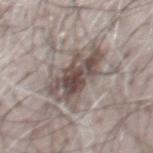• follow-up: catalogued during a skin exam; not biopsied
• patient: male, aged approximately 70
• image: 15 mm crop, total-body photography
• diameter: ~7 mm (longest diameter)
• automated metrics: an average lesion color of about L≈50 a*≈11 b*≈18 (CIELAB); border irregularity of about 4.5 on a 0–10 scale, a color-variation rating of about 8.5/10, and a peripheral color-asymmetry measure near 3
• body site: the chest